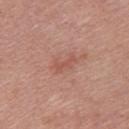The lesion was tiled from a total-body skin photograph and was not biopsied.
Approximately 3 mm at its widest.
A region of skin cropped from a whole-body photographic capture, roughly 15 mm wide.
This is a white-light tile.
The lesion is on the upper back.
The patient is a female in their 40s.